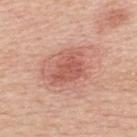Clinical impression: Recorded during total-body skin imaging; not selected for excision or biopsy. Background: A male patient aged 48–52. The lesion is located on the upper back. A 15 mm crop from a total-body photograph taken for skin-cancer surveillance. The tile uses white-light illumination. The lesion's longest dimension is about 4.5 mm.